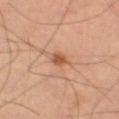The lesion was tiled from a total-body skin photograph and was not biopsied. Longest diameter approximately 2.5 mm. A roughly 15 mm field-of-view crop from a total-body skin photograph. The tile uses cross-polarized illumination. On the left thigh.